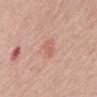– site: the mid back
– patient: male, aged 58 to 62
– acquisition: ~15 mm crop, total-body skin-cancer survey